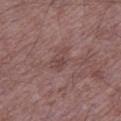follow-up: total-body-photography surveillance lesion; no biopsy | automated metrics: a border-irregularity rating of about 3/10, internal color variation of about 1 on a 0–10 scale, and peripheral color asymmetry of about 0.5 | subject: male, in their 50s | lesion size: ~3 mm (longest diameter) | image: ~15 mm crop, total-body skin-cancer survey | anatomic site: the leg | tile lighting: white-light illumination.